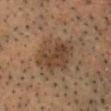Captured during whole-body skin photography for melanoma surveillance; the lesion was not biopsied. The lesion-visualizer software estimated a lesion color around L≈36 a*≈13 b*≈25 in CIELAB, about 7 CIELAB-L* units darker than the surrounding skin, and a lesion-to-skin contrast of about 7 (normalized; higher = more distinct). The analysis additionally found an automated nevus-likeness rating near 10 out of 100 and lesion-presence confidence of about 100/100. Measured at roughly 5.5 mm in maximum diameter. A male patient, aged approximately 55. A roughly 15 mm field-of-view crop from a total-body skin photograph. Imaged with cross-polarized lighting. From the head or neck.